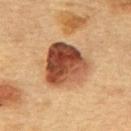{
  "biopsy_status": "not biopsied; imaged during a skin examination",
  "automated_metrics": {
    "border_irregularity_0_10": 2.5,
    "color_variation_0_10": 9.5,
    "nevus_likeness_0_100": 80
  },
  "site": "mid back",
  "patient": {
    "sex": "female",
    "age_approx": 60
  },
  "image": {
    "source": "total-body photography crop",
    "field_of_view_mm": 15
  },
  "lesion_size": {
    "long_diameter_mm_approx": 5.5
  },
  "lighting": "cross-polarized"
}